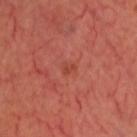biopsy_status: not biopsied; imaged during a skin examination
image:
  source: total-body photography crop
  field_of_view_mm: 15
automated_metrics:
  area_mm2_approx: 1.5
  eccentricity: 0.75
  shape_asymmetry: 0.45
  color_variation_0_10: 0.0
  peripheral_color_asymmetry: 0.0
site: head or neck
lighting: cross-polarized
lesion_size:
  long_diameter_mm_approx: 1.5
patient:
  sex: male
  age_approx: 45